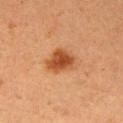* image-analysis metrics: a border-irregularity rating of about 2.5/10, a color-variation rating of about 3/10, and a peripheral color-asymmetry measure near 1; an automated nevus-likeness rating near 100 out of 100 and a lesion-detection confidence of about 100/100
* tile lighting: cross-polarized
* size: about 3.5 mm
* site: the right upper arm
* patient: female, about 40 years old
* image source: ~15 mm crop, total-body skin-cancer survey